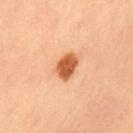follow-up: no biopsy performed (imaged during a skin exam)